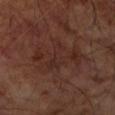Q: Is there a histopathology result?
A: no biopsy performed (imaged during a skin exam)
Q: How was the tile lit?
A: cross-polarized illumination
Q: Lesion location?
A: the left forearm
Q: Lesion size?
A: ≈6.5 mm
Q: How was this image acquired?
A: ~15 mm tile from a whole-body skin photo
Q: What are the patient's age and sex?
A: male, aged 63 to 67